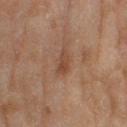<case>
<site>right thigh</site>
<image>
  <source>total-body photography crop</source>
  <field_of_view_mm>15</field_of_view_mm>
</image>
<lighting>cross-polarized</lighting>
<patient>
  <sex>female</sex>
  <age_approx>60</age_approx>
</patient>
<automated_metrics>
  <border_irregularity_0_10>3.5</border_irregularity_0_10>
  <color_variation_0_10>1.5</color_variation_0_10>
  <peripheral_color_asymmetry>0.5</peripheral_color_asymmetry>
</automated_metrics>
<lesion_size>
  <long_diameter_mm_approx>3.0</long_diameter_mm_approx>
</lesion_size>
</case>